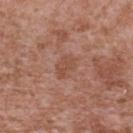biopsy_status: not biopsied; imaged during a skin examination
image:
  source: total-body photography crop
  field_of_view_mm: 15
site: upper back
patient:
  sex: male
  age_approx: 45
automated_metrics:
  area_mm2_approx: 5.5
  eccentricity: 0.7
  shape_asymmetry: 0.25
  nevus_likeness_0_100: 0
  lesion_detection_confidence_0_100: 100